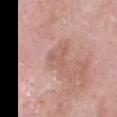{
  "biopsy_status": "not biopsied; imaged during a skin examination"
}